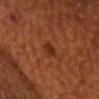Clinical impression: No biopsy was performed on this lesion — it was imaged during a full skin examination and was not determined to be concerning. Image and clinical context: The lesion is on the head or neck. The patient is a male aged approximately 60. The total-body-photography lesion software estimated an automated nevus-likeness rating near 20 out of 100 and a lesion-detection confidence of about 100/100. Approximately 3 mm at its widest. This is a cross-polarized tile. Cropped from a whole-body photographic skin survey; the tile spans about 15 mm.